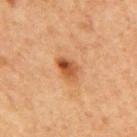workup — total-body-photography surveillance lesion; no biopsy
automated metrics — a footprint of about 5.5 mm², an outline eccentricity of about 0.8 (0 = round, 1 = elongated), and two-axis asymmetry of about 0.2; a mean CIELAB color near L≈46 a*≈23 b*≈36, roughly 11 lightness units darker than nearby skin, and a normalized lesion–skin contrast near 9; a border-irregularity index near 2/10, a within-lesion color-variation index near 8/10, and peripheral color asymmetry of about 3.5; a nevus-likeness score of about 90/100 and lesion-presence confidence of about 100/100
image source — total-body-photography crop, ~15 mm field of view
illumination — cross-polarized
patient — male, aged 63 to 67
size — ≈3.5 mm
body site — the mid back